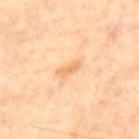Q: Is there a histopathology result?
A: total-body-photography surveillance lesion; no biopsy